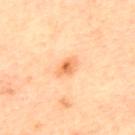Part of a total-body skin-imaging series; this lesion was reviewed on a skin check and was not flagged for biopsy. The patient is a male approximately 70 years of age. Located on the upper back. A 15 mm close-up extracted from a 3D total-body photography capture.